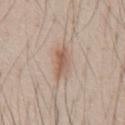Measured at roughly 4.5 mm in maximum diameter. This is a white-light tile. A male patient, aged around 45. On the abdomen. Cropped from a total-body skin-imaging series; the visible field is about 15 mm.